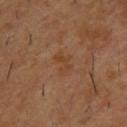biopsy status = imaged on a skin check; not biopsied | subject = male, aged 53–57 | image = ~15 mm tile from a whole-body skin photo | anatomic site = the upper back.